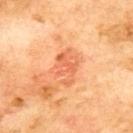Assessment:
Captured during whole-body skin photography for melanoma surveillance; the lesion was not biopsied.
Acquisition and patient details:
Approximately 4.5 mm at its widest. A male patient, aged around 70. An algorithmic analysis of the crop reported a mean CIELAB color near L≈65 a*≈32 b*≈42, roughly 9 lightness units darker than nearby skin, and a lesion-to-skin contrast of about 5.5 (normalized; higher = more distinct). From the upper back. The tile uses cross-polarized illumination. Cropped from a whole-body photographic skin survey; the tile spans about 15 mm.